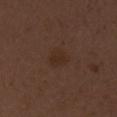workup = catalogued during a skin exam; not biopsied.